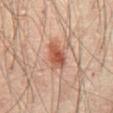Recorded during total-body skin imaging; not selected for excision or biopsy. Imaged with cross-polarized lighting. The subject is a male roughly 65 years of age. About 4 mm across. A 15 mm crop from a total-body photograph taken for skin-cancer surveillance. From the abdomen.